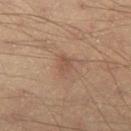follow-up: total-body-photography surveillance lesion; no biopsy | lesion size: about 2.5 mm | subject: male, roughly 40 years of age | image: ~15 mm crop, total-body skin-cancer survey | anatomic site: the left thigh | TBP lesion metrics: a lesion area of about 4 mm², a shape eccentricity near 0.65, and a symmetry-axis asymmetry near 0.35; border irregularity of about 3 on a 0–10 scale and a color-variation rating of about 1.5/10.